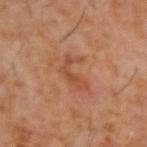| feature | finding |
|---|---|
| site | the upper back |
| lighting | cross-polarized |
| size | ~4 mm (longest diameter) |
| imaging modality | ~15 mm crop, total-body skin-cancer survey |
| subject | male, aged 58–62 |